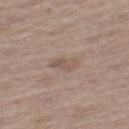anatomic site — the left thigh
imaging modality — ~15 mm tile from a whole-body skin photo
illumination — white-light illumination
patient — male, in their 70s